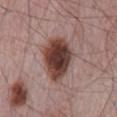Clinical impression:
The lesion was photographed on a routine skin check and not biopsied; there is no pathology result.
Context:
Imaged with white-light lighting. Approximately 6.5 mm at its widest. From the mid back. The patient is a male roughly 65 years of age. Cropped from a total-body skin-imaging series; the visible field is about 15 mm.